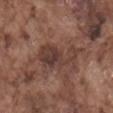Findings:
– anatomic site: the mid back
– automated metrics: a footprint of about 12 mm², an outline eccentricity of about 0.85 (0 = round, 1 = elongated), and two-axis asymmetry of about 0.45; a border-irregularity index near 6.5/10 and internal color variation of about 4 on a 0–10 scale; an automated nevus-likeness rating near 0 out of 100 and a lesion-detection confidence of about 95/100
– acquisition: ~15 mm tile from a whole-body skin photo
– subject: male, in their mid- to late 70s